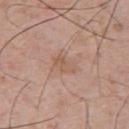Part of a total-body skin-imaging series; this lesion was reviewed on a skin check and was not flagged for biopsy. A male subject approximately 55 years of age. This image is a 15 mm lesion crop taken from a total-body photograph. Imaged with white-light lighting. The lesion is on the upper back. An algorithmic analysis of the crop reported a shape eccentricity near 0.8 and two-axis asymmetry of about 0.25. It also reported an average lesion color of about L≈57 a*≈18 b*≈29 (CIELAB), roughly 6 lightness units darker than nearby skin, and a normalized lesion–skin contrast near 5. And it measured a detector confidence of about 100 out of 100 that the crop contains a lesion.